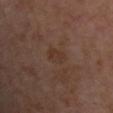subject: about 55 years old | site: the chest | size: ≈3 mm | lighting: cross-polarized | TBP lesion metrics: an area of roughly 5 mm² and an eccentricity of roughly 0.75; a nevus-likeness score of about 0/100 and a detector confidence of about 100 out of 100 that the crop contains a lesion | imaging modality: total-body-photography crop, ~15 mm field of view.